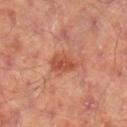workup = catalogued during a skin exam; not biopsied
tile lighting = cross-polarized illumination
patient = male, in their mid-60s
location = the right lower leg
acquisition = 15 mm crop, total-body photography